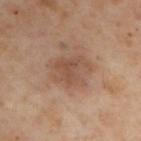The lesion was tiled from a total-body skin photograph and was not biopsied.
The patient is a male aged approximately 55.
On the arm.
This is a cross-polarized tile.
A roughly 15 mm field-of-view crop from a total-body skin photograph.